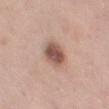No biopsy was performed on this lesion — it was imaged during a full skin examination and was not determined to be concerning. The lesion is on the left thigh. This image is a 15 mm lesion crop taken from a total-body photograph. A female subject aged around 55.